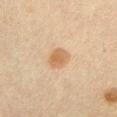biopsy_status: not biopsied; imaged during a skin examination
image:
  source: total-body photography crop
  field_of_view_mm: 15
automated_metrics:
  nevus_likeness_0_100: 90
  lesion_detection_confidence_0_100: 100
lighting: cross-polarized
lesion_size:
  long_diameter_mm_approx: 3.0
site: chest
patient:
  sex: male
  age_approx: 60